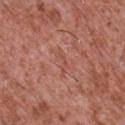Assessment: The lesion was tiled from a total-body skin photograph and was not biopsied. Context: A male subject about 45 years old. Imaged with white-light lighting. The lesion is located on the front of the torso. A 15 mm close-up extracted from a 3D total-body photography capture.